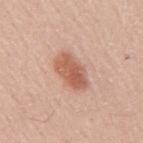A male patient, aged 58–62. A lesion tile, about 15 mm wide, cut from a 3D total-body photograph. Located on the mid back. Automated tile analysis of the lesion measured border irregularity of about 2.5 on a 0–10 scale and radial color variation of about 1.5. The analysis additionally found a classifier nevus-likeness of about 95/100. The lesion's longest dimension is about 4.5 mm.